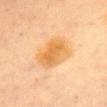Clinical impression:
The lesion was tiled from a total-body skin photograph and was not biopsied.
Background:
The patient is a female approximately 60 years of age. This is a cross-polarized tile. A lesion tile, about 15 mm wide, cut from a 3D total-body photograph. On the chest. The lesion-visualizer software estimated an area of roughly 15 mm². The software also gave an average lesion color of about L≈62 a*≈20 b*≈43 (CIELAB), roughly 8 lightness units darker than nearby skin, and a lesion-to-skin contrast of about 8 (normalized; higher = more distinct). It also reported a border-irregularity rating of about 1.5/10 and a color-variation rating of about 4/10. The analysis additionally found an automated nevus-likeness rating near 85 out of 100 and a detector confidence of about 100 out of 100 that the crop contains a lesion. The lesion's longest dimension is about 5 mm.